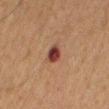This lesion was catalogued during total-body skin photography and was not selected for biopsy.
Located on the mid back.
Cropped from a whole-body photographic skin survey; the tile spans about 15 mm.
An algorithmic analysis of the crop reported a footprint of about 4 mm², an eccentricity of roughly 0.7, and two-axis asymmetry of about 0.15. The software also gave an average lesion color of about L≈33 a*≈22 b*≈23 (CIELAB) and roughly 13 lightness units darker than nearby skin.
The subject is a male about 65 years old.
The recorded lesion diameter is about 2.5 mm.
This is a cross-polarized tile.